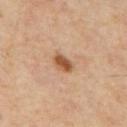The lesion was photographed on a routine skin check and not biopsied; there is no pathology result. From the mid back. A 15 mm close-up tile from a total-body photography series done for melanoma screening. Automated tile analysis of the lesion measured an area of roughly 4 mm², an outline eccentricity of about 0.8 (0 = round, 1 = elongated), and a symmetry-axis asymmetry near 0.25. And it measured a lesion-to-skin contrast of about 9.5 (normalized; higher = more distinct). It also reported a border-irregularity index near 2.5/10 and peripheral color asymmetry of about 1.5. The lesion's longest dimension is about 2.5 mm. Captured under cross-polarized illumination. The subject is a male in their mid-50s.